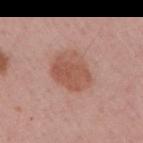Impression:
Part of a total-body skin-imaging series; this lesion was reviewed on a skin check and was not flagged for biopsy.
Context:
A roughly 15 mm field-of-view crop from a total-body skin photograph. Approximately 5 mm at its widest. The lesion is on the right upper arm. This is a white-light tile. The subject is a female aged 53–57. The lesion-visualizer software estimated an eccentricity of roughly 0.65 and a symmetry-axis asymmetry near 0.15. It also reported a border-irregularity rating of about 1.5/10, a color-variation rating of about 3.5/10, and a peripheral color-asymmetry measure near 1. It also reported an automated nevus-likeness rating near 20 out of 100 and a detector confidence of about 100 out of 100 that the crop contains a lesion.